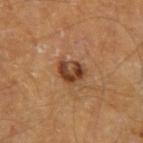biopsy_status: not biopsied; imaged during a skin examination
automated_metrics:
  nevus_likeness_0_100: 85
  lesion_detection_confidence_0_100: 100
lesion_size:
  long_diameter_mm_approx: 3.0
site: left forearm
lighting: cross-polarized
image:
  source: total-body photography crop
  field_of_view_mm: 15
patient:
  sex: male
  age_approx: 70